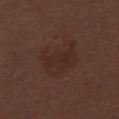biopsy status=catalogued during a skin exam; not biopsied
image-analysis metrics=a symmetry-axis asymmetry near 0.3
subject=female, aged 28–32
tile lighting=white-light illumination
site=the right lower leg
image=~15 mm tile from a whole-body skin photo
lesion size=≈5 mm